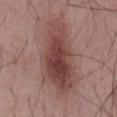Findings:
* follow-up: imaged on a skin check; not biopsied